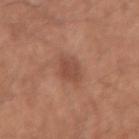Impression:
Recorded during total-body skin imaging; not selected for excision or biopsy.
Background:
Approximately 3 mm at its widest. Automated tile analysis of the lesion measured a mean CIELAB color near L≈48 a*≈24 b*≈29, a lesion–skin lightness drop of about 8, and a normalized border contrast of about 6. And it measured a border-irregularity index near 1.5/10 and peripheral color asymmetry of about 0.5. The tile uses white-light illumination. A 15 mm close-up extracted from a 3D total-body photography capture. The patient is a male aged 53 to 57. The lesion is located on the right upper arm.